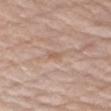{"biopsy_status": "not biopsied; imaged during a skin examination", "image": {"source": "total-body photography crop", "field_of_view_mm": 15}, "lighting": "white-light", "patient": {"sex": "male", "age_approx": 75}, "site": "right upper arm", "lesion_size": {"long_diameter_mm_approx": 2.5}}